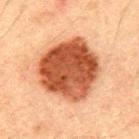Imaged during a routine full-body skin examination; the lesion was not biopsied and no histopathology is available.
A roughly 15 mm field-of-view crop from a total-body skin photograph.
From the mid back.
The lesion-visualizer software estimated a lesion area of about 38 mm², an outline eccentricity of about 0.35 (0 = round, 1 = elongated), and two-axis asymmetry of about 0.15.
The tile uses cross-polarized illumination.
A male patient, in their 50s.